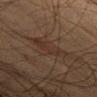Clinical summary:
Located on the abdomen. Captured under cross-polarized illumination. The subject is a male roughly 60 years of age. A 15 mm close-up tile from a total-body photography series done for melanoma screening.